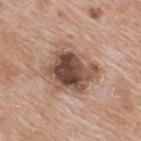Background: A lesion tile, about 15 mm wide, cut from a 3D total-body photograph. The tile uses white-light illumination. Located on the back. A male patient approximately 65 years of age. The lesion-visualizer software estimated a mean CIELAB color near L≈50 a*≈18 b*≈26, a lesion–skin lightness drop of about 15, and a normalized border contrast of about 10.5. The software also gave a nevus-likeness score of about 10/100 and lesion-presence confidence of about 100/100. About 5.5 mm across.